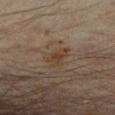Measured at roughly 3.5 mm in maximum diameter.
A male patient aged 43 to 47.
A 15 mm close-up tile from a total-body photography series done for melanoma screening.
On the right forearm.